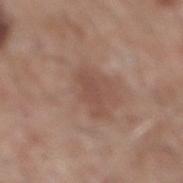Q: Is there a histopathology result?
A: total-body-photography surveillance lesion; no biopsy
Q: Lesion location?
A: the mid back
Q: What are the patient's age and sex?
A: male, aged 58 to 62
Q: Automated lesion metrics?
A: about 7 CIELAB-L* units darker than the surrounding skin and a lesion-to-skin contrast of about 5.5 (normalized; higher = more distinct); a border-irregularity index near 4.5/10, a color-variation rating of about 0.5/10, and radial color variation of about 0
Q: What kind of image is this?
A: ~15 mm tile from a whole-body skin photo
Q: What lighting was used for the tile?
A: white-light illumination
Q: What is the lesion's diameter?
A: ~3.5 mm (longest diameter)